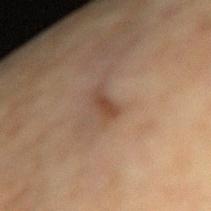Notes:
- follow-up · no biopsy performed (imaged during a skin exam)
- automated metrics · a footprint of about 1.5 mm²; roughly 9 lightness units darker than nearby skin and a normalized border contrast of about 8.5
- image source · 15 mm crop, total-body photography
- illumination · cross-polarized
- size · about 2.5 mm
- location · the right upper arm
- subject · female, in their 80s